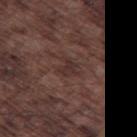biopsy status=no biopsy performed (imaged during a skin exam); lesion size=≈2.5 mm; tile lighting=white-light illumination; body site=the left thigh; subject=male, aged approximately 75; image source=~15 mm tile from a whole-body skin photo.